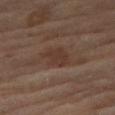lighting = cross-polarized; patient = female, roughly 70 years of age; site = the right thigh; acquisition = total-body-photography crop, ~15 mm field of view; size = about 3 mm.